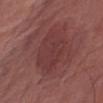No biopsy was performed on this lesion — it was imaged during a full skin examination and was not determined to be concerning.